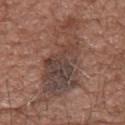Clinical impression:
No biopsy was performed on this lesion — it was imaged during a full skin examination and was not determined to be concerning.
Image and clinical context:
This image is a 15 mm lesion crop taken from a total-body photograph. Located on the upper back. A male subject about 75 years old.